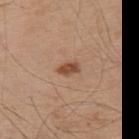Captured during whole-body skin photography for melanoma surveillance; the lesion was not biopsied. On the upper back. A male patient in their mid- to late 50s. The tile uses white-light illumination. A roughly 15 mm field-of-view crop from a total-body skin photograph. Automated tile analysis of the lesion measured a footprint of about 3.5 mm² and an outline eccentricity of about 0.8 (0 = round, 1 = elongated). The analysis additionally found a border-irregularity rating of about 2/10. Measured at roughly 2.5 mm in maximum diameter.